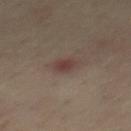follow-up: catalogued during a skin exam; not biopsied | patient: male, aged 43 to 47 | size: ≈2.5 mm | tile lighting: cross-polarized illumination | imaging modality: ~15 mm tile from a whole-body skin photo | body site: the mid back.